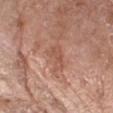Part of a total-body skin-imaging series; this lesion was reviewed on a skin check and was not flagged for biopsy.
The lesion's longest dimension is about 3.5 mm.
A region of skin cropped from a whole-body photographic capture, roughly 15 mm wide.
The tile uses white-light illumination.
Located on the arm.
The subject is a female about 75 years old.
Automated tile analysis of the lesion measured a lesion area of about 5 mm², an eccentricity of roughly 0.85, and a symmetry-axis asymmetry near 0.35. The analysis additionally found border irregularity of about 4.5 on a 0–10 scale. And it measured a detector confidence of about 100 out of 100 that the crop contains a lesion.